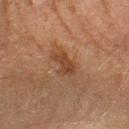Q: Was this lesion biopsied?
A: total-body-photography surveillance lesion; no biopsy
Q: What is the anatomic site?
A: the left forearm
Q: What lighting was used for the tile?
A: cross-polarized illumination
Q: What is the lesion's diameter?
A: about 3 mm
Q: Who is the patient?
A: male, in their mid-60s
Q: What did automated image analysis measure?
A: a shape eccentricity near 0.75 and a shape-asymmetry score of about 0.4 (0 = symmetric); an average lesion color of about L≈34 a*≈18 b*≈27 (CIELAB) and a lesion–skin lightness drop of about 7
Q: How was this image acquired?
A: ~15 mm tile from a whole-body skin photo